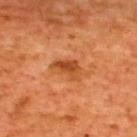  biopsy_status: not biopsied; imaged during a skin examination
  patient:
    sex: male
    age_approx: 65
  image:
    source: total-body photography crop
    field_of_view_mm: 15
  site: upper back